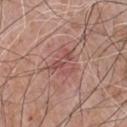Q: Was this lesion biopsied?
A: catalogued during a skin exam; not biopsied
Q: Lesion location?
A: the front of the torso
Q: Who is the patient?
A: male, in their mid- to late 60s
Q: How was this image acquired?
A: ~15 mm crop, total-body skin-cancer survey
Q: Illumination type?
A: white-light